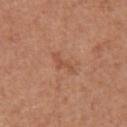This is a white-light tile. Longest diameter approximately 3 mm. A male subject, roughly 65 years of age. A lesion tile, about 15 mm wide, cut from a 3D total-body photograph. Located on the abdomen. Automated image analysis of the tile measured an area of roughly 3 mm² and a shape-asymmetry score of about 0.65 (0 = symmetric). It also reported a border-irregularity rating of about 7/10, a within-lesion color-variation index near 0/10, and radial color variation of about 0. The analysis additionally found a classifier nevus-likeness of about 0/100 and a lesion-detection confidence of about 100/100.